This image is a 15 mm lesion crop taken from a total-body photograph; the recorded lesion diameter is about 8 mm; the tile uses white-light illumination; located on the chest; the patient is a male approximately 60 years of age: 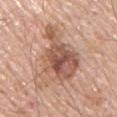On biopsy, histopathology showed an invasive melanoma arising in association with a nevus (Breslow thickness 0.3 mm, mitotic rate <1/mm²) — a malignant skin lesion.